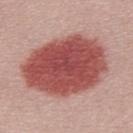workup = catalogued during a skin exam; not biopsied
automated metrics = an average lesion color of about L≈50 a*≈30 b*≈25 (CIELAB) and roughly 18 lightness units darker than nearby skin; border irregularity of about 1.5 on a 0–10 scale and a color-variation rating of about 4.5/10; a classifier nevus-likeness of about 100/100 and a detector confidence of about 100 out of 100 that the crop contains a lesion
illumination = white-light
subject = male, approximately 30 years of age
acquisition = 15 mm crop, total-body photography